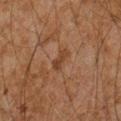Q: Was a biopsy performed?
A: catalogued during a skin exam; not biopsied
Q: Who is the patient?
A: male, aged around 45
Q: How was the tile lit?
A: cross-polarized
Q: Where on the body is the lesion?
A: the right forearm
Q: What is the imaging modality?
A: ~15 mm crop, total-body skin-cancer survey
Q: Automated lesion metrics?
A: an area of roughly 3.5 mm² and an outline eccentricity of about 0.85 (0 = round, 1 = elongated); a lesion color around L≈33 a*≈18 b*≈27 in CIELAB and a lesion–skin lightness drop of about 6
Q: How large is the lesion?
A: ≈3 mm